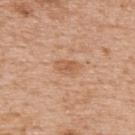biopsy_status: not biopsied; imaged during a skin examination
lighting: white-light
image:
  source: total-body photography crop
  field_of_view_mm: 15
site: upper back
automated_metrics:
  cielab_L: 58
  cielab_a: 22
  cielab_b: 35
  vs_skin_darker_L: 8.0
  vs_skin_contrast_norm: 5.5
patient:
  sex: male
  age_approx: 65